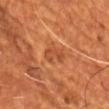Imaged during a routine full-body skin examination; the lesion was not biopsied and no histopathology is available. The recorded lesion diameter is about 3 mm. Captured under cross-polarized illumination. Cropped from a total-body skin-imaging series; the visible field is about 15 mm. On the front of the torso. A male patient, aged around 55.